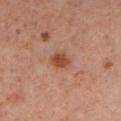workup — catalogued during a skin exam; not biopsied
site — the left lower leg
image source — ~15 mm crop, total-body skin-cancer survey
tile lighting — cross-polarized illumination
lesion size — ≈2.5 mm
patient — male, aged 58 to 62
automated lesion analysis — a classifier nevus-likeness of about 60/100 and a lesion-detection confidence of about 100/100